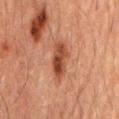Findings:
• biopsy status · total-body-photography surveillance lesion; no biopsy
• automated metrics · a footprint of about 8 mm² and an outline eccentricity of about 0.9 (0 = round, 1 = elongated); a classifier nevus-likeness of about 80/100 and lesion-presence confidence of about 100/100
• illumination · cross-polarized illumination
• body site · the mid back
• subject · male, aged approximately 60
• image source · total-body-photography crop, ~15 mm field of view